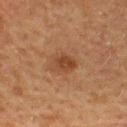<case>
  <image>
    <source>total-body photography crop</source>
    <field_of_view_mm>15</field_of_view_mm>
  </image>
  <patient>
    <sex>female</sex>
    <age_approx>55</age_approx>
  </patient>
  <site>upper back</site>
</case>